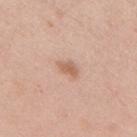- notes: no biopsy performed (imaged during a skin exam)
- lighting: white-light
- lesion diameter: about 2.5 mm
- site: the back
- subject: male, aged around 40
- acquisition: 15 mm crop, total-body photography